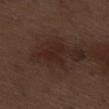The lesion was tiled from a total-body skin photograph and was not biopsied.
The tile uses white-light illumination.
Measured at roughly 3 mm in maximum diameter.
The lesion is on the right thigh.
A lesion tile, about 15 mm wide, cut from a 3D total-body photograph.
A male subject in their 70s.